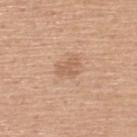{"biopsy_status": "not biopsied; imaged during a skin examination", "lighting": "white-light", "lesion_size": {"long_diameter_mm_approx": 2.5}, "site": "back", "patient": {"sex": "female", "age_approx": 60}, "image": {"source": "total-body photography crop", "field_of_view_mm": 15}, "automated_metrics": {"cielab_L": 60, "cielab_a": 20, "cielab_b": 32, "vs_skin_darker_L": 8.0, "vs_skin_contrast_norm": 5.5, "color_variation_0_10": 0.0, "peripheral_color_asymmetry": 0.0, "nevus_likeness_0_100": 0, "lesion_detection_confidence_0_100": 100}}